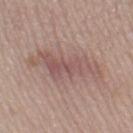Case summary:
* biopsy status — imaged on a skin check; not biopsied
* patient — female, in their 60s
* image — ~15 mm tile from a whole-body skin photo
* location — the left thigh